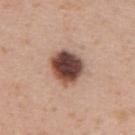Assessment: Captured during whole-body skin photography for melanoma surveillance; the lesion was not biopsied. Clinical summary: The lesion-visualizer software estimated internal color variation of about 8 on a 0–10 scale. The software also gave an automated nevus-likeness rating near 60 out of 100 and lesion-presence confidence of about 100/100. A lesion tile, about 15 mm wide, cut from a 3D total-body photograph. The subject is a female aged 28–32. On the upper back. Imaged with white-light lighting.